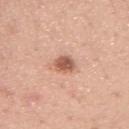No biopsy was performed on this lesion — it was imaged during a full skin examination and was not determined to be concerning. Imaged with white-light lighting. A 15 mm close-up extracted from a 3D total-body photography capture. The lesion-visualizer software estimated a lesion area of about 4.5 mm², a shape eccentricity near 0.65, and a shape-asymmetry score of about 0.25 (0 = symmetric). It also reported a lesion–skin lightness drop of about 15 and a normalized lesion–skin contrast near 9. And it measured a border-irregularity rating of about 2.5/10 and radial color variation of about 1. The patient is a female in their mid- to late 30s. On the left upper arm. Approximately 2.5 mm at its widest.